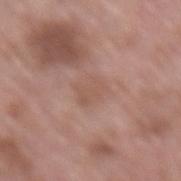Impression: The lesion was tiled from a total-body skin photograph and was not biopsied. Context: The lesion is on the lower back. A male patient aged around 55. A roughly 15 mm field-of-view crop from a total-body skin photograph.